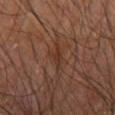Part of a total-body skin-imaging series; this lesion was reviewed on a skin check and was not flagged for biopsy.